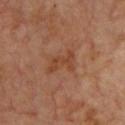Captured during whole-body skin photography for melanoma surveillance; the lesion was not biopsied. A subject in their mid-60s. Automated tile analysis of the lesion measured an average lesion color of about L≈42 a*≈23 b*≈32 (CIELAB) and a lesion–skin lightness drop of about 7. It also reported a border-irregularity index near 5.5/10, internal color variation of about 1.5 on a 0–10 scale, and radial color variation of about 0.5. And it measured a classifier nevus-likeness of about 0/100. A region of skin cropped from a whole-body photographic capture, roughly 15 mm wide. Approximately 4 mm at its widest. On the chest. Imaged with cross-polarized lighting.